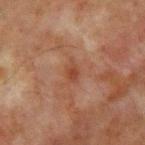Assessment: No biopsy was performed on this lesion — it was imaged during a full skin examination and was not determined to be concerning. Clinical summary: The patient is a male about 65 years old. A 15 mm close-up tile from a total-body photography series done for melanoma screening. On the chest. Approximately 2.5 mm at its widest.